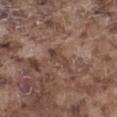| key | value |
|---|---|
| biopsy status | imaged on a skin check; not biopsied |
| image source | ~15 mm crop, total-body skin-cancer survey |
| subject | male, in their mid- to late 70s |
| lesion size | ~3.5 mm (longest diameter) |
| automated metrics | a shape-asymmetry score of about 0.35 (0 = symmetric); a nevus-likeness score of about 0/100 |
| body site | the left thigh |